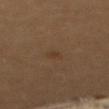Impression:
Captured during whole-body skin photography for melanoma surveillance; the lesion was not biopsied.